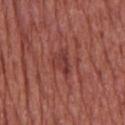Imaged during a routine full-body skin examination; the lesion was not biopsied and no histopathology is available. Cropped from a whole-body photographic skin survey; the tile spans about 15 mm. From the chest. An algorithmic analysis of the crop reported an average lesion color of about L≈39 a*≈28 b*≈25 (CIELAB), about 7 CIELAB-L* units darker than the surrounding skin, and a normalized lesion–skin contrast near 6.5. The software also gave border irregularity of about 5 on a 0–10 scale. And it measured an automated nevus-likeness rating near 0 out of 100. A male subject, aged 63–67. Approximately 3 mm at its widest.